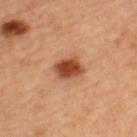This lesion was catalogued during total-body skin photography and was not selected for biopsy.
The subject is a female in their mid- to late 40s.
A region of skin cropped from a whole-body photographic capture, roughly 15 mm wide.
On the left upper arm.